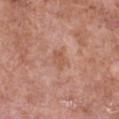Impression:
This lesion was catalogued during total-body skin photography and was not selected for biopsy.
Background:
A 15 mm close-up extracted from a 3D total-body photography capture. Automated tile analysis of the lesion measured an eccentricity of roughly 0.7 and a symmetry-axis asymmetry near 0.25. It also reported a nevus-likeness score of about 0/100. A female patient, aged 73–77. The tile uses white-light illumination. Located on the chest.